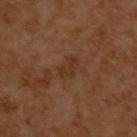biopsy status=imaged on a skin check; not biopsied
size=~3 mm (longest diameter)
image=~15 mm crop, total-body skin-cancer survey
location=the back
lighting=cross-polarized
subject=male, roughly 60 years of age